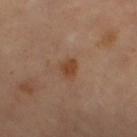Imaged during a routine full-body skin examination; the lesion was not biopsied and no histopathology is available. From the left thigh. A female patient roughly 70 years of age. A lesion tile, about 15 mm wide, cut from a 3D total-body photograph.